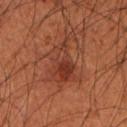Case summary:
- biopsy status · total-body-photography surveillance lesion; no biopsy